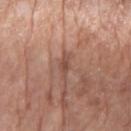  biopsy_status: not biopsied; imaged during a skin examination
  patient:
    sex: female
    age_approx: 75
  image:
    source: total-body photography crop
    field_of_view_mm: 15
  lesion_size:
    long_diameter_mm_approx: 3.0
  site: left forearm
  lighting: white-light